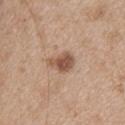Assessment:
This lesion was catalogued during total-body skin photography and was not selected for biopsy.
Context:
A female subject, in their mid- to late 40s. The lesion-visualizer software estimated a lesion–skin lightness drop of about 13 and a lesion-to-skin contrast of about 8.5 (normalized; higher = more distinct). The software also gave an automated nevus-likeness rating near 90 out of 100. Imaged with white-light lighting. A 15 mm close-up tile from a total-body photography series done for melanoma screening. Located on the upper back.